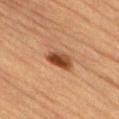Notes:
- anatomic site — the lower back
- patient — male, aged 83 or over
- image source — ~15 mm crop, total-body skin-cancer survey
- lighting — cross-polarized
- diameter — about 3 mm